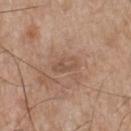This lesion was catalogued during total-body skin photography and was not selected for biopsy. Captured under white-light illumination. A 15 mm close-up tile from a total-body photography series done for melanoma screening. From the chest. Approximately 3.5 mm at its widest. A male subject in their mid- to late 60s.